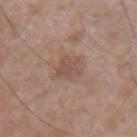Q: Was a biopsy performed?
A: imaged on a skin check; not biopsied
Q: Illumination type?
A: white-light illumination
Q: Where on the body is the lesion?
A: the left upper arm
Q: Who is the patient?
A: male, approximately 50 years of age
Q: How large is the lesion?
A: ~3 mm (longest diameter)
Q: How was this image acquired?
A: 15 mm crop, total-body photography